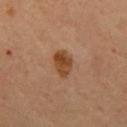Q: Was a biopsy performed?
A: no biopsy performed (imaged during a skin exam)
Q: What is the lesion's diameter?
A: ≈3.5 mm
Q: Illumination type?
A: cross-polarized illumination
Q: What are the patient's age and sex?
A: male, approximately 55 years of age
Q: What kind of image is this?
A: 15 mm crop, total-body photography
Q: Where on the body is the lesion?
A: the mid back